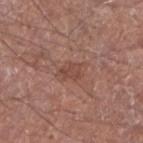A male patient, in their mid- to late 60s. From the right forearm. A 15 mm close-up extracted from a 3D total-body photography capture. Imaged with white-light lighting. Longest diameter approximately 2.5 mm.